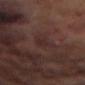The lesion was photographed on a routine skin check and not biopsied; there is no pathology result. The total-body-photography lesion software estimated a lesion area of about 4.5 mm², a shape eccentricity near 0.8, and a shape-asymmetry score of about 0.3 (0 = symmetric). The software also gave an average lesion color of about L≈24 a*≈15 b*≈15 (CIELAB), a lesion–skin lightness drop of about 4, and a normalized lesion–skin contrast near 5. It also reported an automated nevus-likeness rating near 0 out of 100 and a detector confidence of about 70 out of 100 that the crop contains a lesion. Approximately 3 mm at its widest. A female patient, approximately 80 years of age. A 15 mm close-up tile from a total-body photography series done for melanoma screening. On the abdomen.